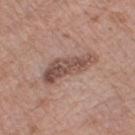Impression: Recorded during total-body skin imaging; not selected for excision or biopsy. Background: On the left thigh. A 15 mm close-up tile from a total-body photography series done for melanoma screening. This is a white-light tile. Longest diameter approximately 6.5 mm. A female subject aged around 75.